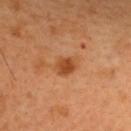- workup · total-body-photography surveillance lesion; no biopsy
- lighting · cross-polarized
- automated lesion analysis · a shape eccentricity near 0.6 and a symmetry-axis asymmetry near 0.25
- body site · the upper back
- diameter · ≈3 mm
- acquisition · total-body-photography crop, ~15 mm field of view
- patient · female, approximately 35 years of age